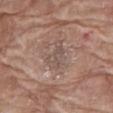Impression:
The lesion was tiled from a total-body skin photograph and was not biopsied.
Clinical summary:
The tile uses white-light illumination. The lesion is on the right thigh. A 15 mm close-up tile from a total-body photography series done for melanoma screening. The total-body-photography lesion software estimated a mean CIELAB color near L≈51 a*≈15 b*≈22 and a lesion-to-skin contrast of about 5 (normalized; higher = more distinct). And it measured border irregularity of about 4.5 on a 0–10 scale, internal color variation of about 3 on a 0–10 scale, and peripheral color asymmetry of about 1. And it measured an automated nevus-likeness rating near 0 out of 100. A female patient aged 73 to 77. The recorded lesion diameter is about 3.5 mm.